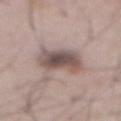Assessment:
Imaged during a routine full-body skin examination; the lesion was not biopsied and no histopathology is available.
Context:
The lesion's longest dimension is about 5 mm. A close-up tile cropped from a whole-body skin photograph, about 15 mm across. Captured under white-light illumination. The lesion is located on the abdomen. A male subject, aged 53–57. The lesion-visualizer software estimated a border-irregularity rating of about 2.5/10, internal color variation of about 6 on a 0–10 scale, and a peripheral color-asymmetry measure near 1.5. It also reported a nevus-likeness score of about 50/100 and a lesion-detection confidence of about 100/100.